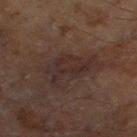Context:
From the left thigh. Approximately 6 mm at its widest. A roughly 15 mm field-of-view crop from a total-body skin photograph. Automated image analysis of the tile measured a footprint of about 14 mm², an outline eccentricity of about 0.8 (0 = round, 1 = elongated), and a shape-asymmetry score of about 0.35 (0 = symmetric). The software also gave an average lesion color of about L≈22 a*≈12 b*≈15 (CIELAB), about 5 CIELAB-L* units darker than the surrounding skin, and a lesion-to-skin contrast of about 6 (normalized; higher = more distinct). The software also gave a lesion-detection confidence of about 95/100. A male patient, about 70 years old. The tile uses cross-polarized illumination.